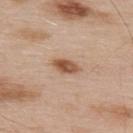biopsy status — imaged on a skin check; not biopsied
diameter — ≈3.5 mm
subject — male, about 55 years old
anatomic site — the upper back
TBP lesion metrics — a footprint of about 5.5 mm², an outline eccentricity of about 0.85 (0 = round, 1 = elongated), and two-axis asymmetry of about 0.2
acquisition — ~15 mm crop, total-body skin-cancer survey